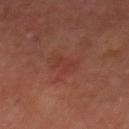Part of a total-body skin-imaging series; this lesion was reviewed on a skin check and was not flagged for biopsy. The lesion-visualizer software estimated a lesion area of about 4.5 mm², an outline eccentricity of about 0.8 (0 = round, 1 = elongated), and two-axis asymmetry of about 0.3. And it measured about 4 CIELAB-L* units darker than the surrounding skin and a normalized border contrast of about 4. It also reported internal color variation of about 1 on a 0–10 scale and radial color variation of about 0.5. On the abdomen. A male patient, approximately 65 years of age. Cropped from a whole-body photographic skin survey; the tile spans about 15 mm. Captured under cross-polarized illumination.